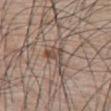notes: no biopsy performed (imaged during a skin exam) | location: the chest | lesion size: ≈3.5 mm | acquisition: total-body-photography crop, ~15 mm field of view | patient: male, approximately 75 years of age | tile lighting: white-light.